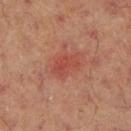{
  "biopsy_status": "not biopsied; imaged during a skin examination",
  "lesion_size": {
    "long_diameter_mm_approx": 3.5
  },
  "image": {
    "source": "total-body photography crop",
    "field_of_view_mm": 15
  },
  "site": "right lower leg",
  "lighting": "cross-polarized",
  "automated_metrics": {
    "area_mm2_approx": 6.0,
    "shape_asymmetry": 0.35,
    "border_irregularity_0_10": 4.0,
    "nevus_likeness_0_100": 0,
    "lesion_detection_confidence_0_100": 100
  },
  "patient": {
    "sex": "male",
    "age_approx": 70
  }
}